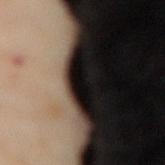{"biopsy_status": "not biopsied; imaged during a skin examination", "patient": {"sex": "female", "age_approx": 55}, "lesion_size": {"long_diameter_mm_approx": 5.0}, "automated_metrics": {"eccentricity": 0.95, "shape_asymmetry": 0.45, "nevus_likeness_0_100": 15}, "image": {"source": "total-body photography crop", "field_of_view_mm": 15}, "lighting": "cross-polarized", "site": "back"}